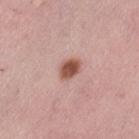  biopsy_status: not biopsied; imaged during a skin examination
  site: left thigh
  patient:
    sex: female
    age_approx: 45
  image:
    source: total-body photography crop
    field_of_view_mm: 15